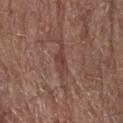biopsy_status: not biopsied; imaged during a skin examination
lighting: white-light
image:
  source: total-body photography crop
  field_of_view_mm: 15
patient:
  sex: female
  age_approx: 80
site: right forearm
lesion_size:
  long_diameter_mm_approx: 3.0